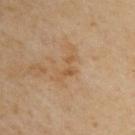Findings:
– biopsy status: imaged on a skin check; not biopsied
– patient: male, aged 53 to 57
– imaging modality: ~15 mm crop, total-body skin-cancer survey
– automated lesion analysis: an area of roughly 4.5 mm², an outline eccentricity of about 0.9 (0 = round, 1 = elongated), and two-axis asymmetry of about 0.6; a lesion color around L≈57 a*≈18 b*≈37 in CIELAB, a lesion–skin lightness drop of about 6, and a lesion-to-skin contrast of about 5.5 (normalized; higher = more distinct); a detector confidence of about 100 out of 100 that the crop contains a lesion
– lighting: cross-polarized
– location: the upper back